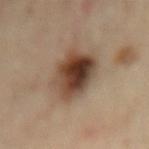Q: Was this lesion biopsied?
A: imaged on a skin check; not biopsied
Q: Patient demographics?
A: female, aged 58–62
Q: What is the anatomic site?
A: the mid back
Q: What did automated image analysis measure?
A: a classifier nevus-likeness of about 95/100 and a lesion-detection confidence of about 100/100
Q: What is the imaging modality?
A: ~15 mm crop, total-body skin-cancer survey
Q: Lesion size?
A: ~6 mm (longest diameter)
Q: Illumination type?
A: cross-polarized